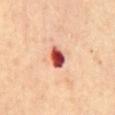- follow-up · no biopsy performed (imaged during a skin exam)
- subject · female, in their mid- to late 60s
- tile lighting · cross-polarized illumination
- lesion diameter · about 3 mm
- acquisition · 15 mm crop, total-body photography
- location · the back
- image-analysis metrics · a border-irregularity rating of about 2/10, internal color variation of about 8 on a 0–10 scale, and peripheral color asymmetry of about 2.5